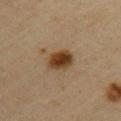This lesion was catalogued during total-body skin photography and was not selected for biopsy.
The subject is a female aged 43–47.
Cropped from a whole-body photographic skin survey; the tile spans about 15 mm.
The lesion is located on the left upper arm.